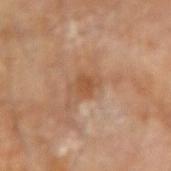| key | value |
|---|---|
| follow-up | no biopsy performed (imaged during a skin exam) |
| anatomic site | the left forearm |
| image | ~15 mm tile from a whole-body skin photo |
| TBP lesion metrics | an area of roughly 4 mm², an outline eccentricity of about 0.65 (0 = round, 1 = elongated), and a symmetry-axis asymmetry near 0.3; a lesion color around L≈49 a*≈21 b*≈34 in CIELAB, roughly 8 lightness units darker than nearby skin, and a normalized lesion–skin contrast near 6.5 |
| patient | male, about 85 years old or older |
| lesion size | ≈3 mm |
| illumination | cross-polarized |